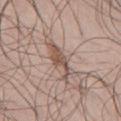Assessment: This lesion was catalogued during total-body skin photography and was not selected for biopsy. Acquisition and patient details: A lesion tile, about 15 mm wide, cut from a 3D total-body photograph. Located on the abdomen. The subject is a male aged 43–47.